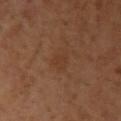Clinical impression: Part of a total-body skin-imaging series; this lesion was reviewed on a skin check and was not flagged for biopsy. Image and clinical context: A 15 mm close-up extracted from a 3D total-body photography capture. This is a cross-polarized tile. The lesion is on the right upper arm. A female subject aged around 40. The lesion-visualizer software estimated a lesion area of about 3.5 mm², a shape eccentricity near 0.75, and a shape-asymmetry score of about 0.3 (0 = symmetric). The analysis additionally found a lesion color around L≈36 a*≈20 b*≈31 in CIELAB and a normalized lesion–skin contrast near 4.5. And it measured an automated nevus-likeness rating near 0 out of 100 and a lesion-detection confidence of about 100/100.